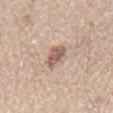Acquisition and patient details:
The recorded lesion diameter is about 3 mm. A 15 mm crop from a total-body photograph taken for skin-cancer surveillance. The subject is a male in their mid- to late 60s. From the chest. An algorithmic analysis of the crop reported a border-irregularity rating of about 2.5/10, a within-lesion color-variation index near 2/10, and peripheral color asymmetry of about 1.